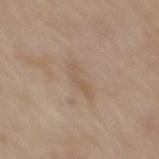Part of a total-body skin-imaging series; this lesion was reviewed on a skin check and was not flagged for biopsy. A male patient aged 78–82. Cropped from a total-body skin-imaging series; the visible field is about 15 mm. Located on the back. Imaged with white-light lighting. Measured at roughly 3.5 mm in maximum diameter.